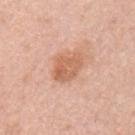The lesion was photographed on a routine skin check and not biopsied; there is no pathology result. This is a white-light tile. An algorithmic analysis of the crop reported a lesion color around L≈62 a*≈24 b*≈33 in CIELAB, a lesion–skin lightness drop of about 10, and a normalized border contrast of about 7. The analysis additionally found a border-irregularity rating of about 2.5/10 and peripheral color asymmetry of about 1.5. It also reported a nevus-likeness score of about 35/100. On the chest. A male subject, roughly 45 years of age. A close-up tile cropped from a whole-body skin photograph, about 15 mm across.